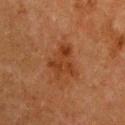{
  "biopsy_status": "not biopsied; imaged during a skin examination",
  "patient": {
    "sex": "female",
    "age_approx": 50
  },
  "site": "chest",
  "lesion_size": {
    "long_diameter_mm_approx": 4.0
  },
  "lighting": "cross-polarized",
  "image": {
    "source": "total-body photography crop",
    "field_of_view_mm": 15
  }
}